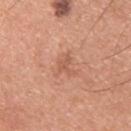- follow-up — no biopsy performed (imaged during a skin exam)
- site — the upper back
- imaging modality — total-body-photography crop, ~15 mm field of view
- patient — male, approximately 30 years of age